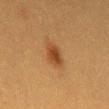follow-up: total-body-photography surveillance lesion; no biopsy
image source: ~15 mm tile from a whole-body skin photo
patient: female, aged around 40
site: the mid back
lighting: cross-polarized illumination
lesion diameter: about 3 mm
TBP lesion metrics: an area of roughly 5.5 mm² and two-axis asymmetry of about 0.2; a lesion color around L≈37 a*≈20 b*≈33 in CIELAB, a lesion–skin lightness drop of about 9, and a lesion-to-skin contrast of about 8 (normalized; higher = more distinct); a classifier nevus-likeness of about 100/100 and a detector confidence of about 100 out of 100 that the crop contains a lesion